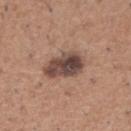lesion diameter = ~4.5 mm (longest diameter) | lighting = white-light | location = the right upper arm | acquisition = ~15 mm crop, total-body skin-cancer survey | subject = male, approximately 50 years of age.